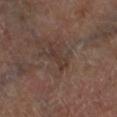The lesion was photographed on a routine skin check and not biopsied; there is no pathology result. A 15 mm close-up tile from a total-body photography series done for melanoma screening. Located on the left lower leg. A male subject, approximately 70 years of age. The total-body-photography lesion software estimated a border-irregularity rating of about 4/10 and radial color variation of about 0. And it measured a nevus-likeness score of about 0/100 and a detector confidence of about 90 out of 100 that the crop contains a lesion.